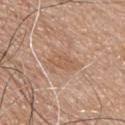Part of a total-body skin-imaging series; this lesion was reviewed on a skin check and was not flagged for biopsy.
A male patient aged around 75.
A 15 mm close-up tile from a total-body photography series done for melanoma screening.
Located on the upper back.